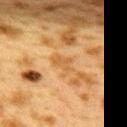biopsy status: no biopsy performed (imaged during a skin exam)
size: about 3 mm
image: total-body-photography crop, ~15 mm field of view
anatomic site: the upper back
automated lesion analysis: an area of roughly 3.5 mm², a shape eccentricity near 0.8, and a symmetry-axis asymmetry near 0.35
lighting: cross-polarized
subject: female, roughly 40 years of age